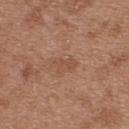follow-up = catalogued during a skin exam; not biopsied
image source = ~15 mm crop, total-body skin-cancer survey
lesion size = ≈3.5 mm
subject = female, roughly 40 years of age
site = the upper back
automated lesion analysis = an automated nevus-likeness rating near 0 out of 100 and a lesion-detection confidence of about 100/100